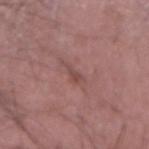follow-up: catalogued during a skin exam; not biopsied | illumination: white-light | body site: the left forearm | acquisition: total-body-photography crop, ~15 mm field of view | subject: male, about 50 years old | automated lesion analysis: an area of roughly 2.5 mm² and a shape eccentricity near 0.9; border irregularity of about 5 on a 0–10 scale, internal color variation of about 0 on a 0–10 scale, and a peripheral color-asymmetry measure near 0; an automated nevus-likeness rating near 0 out of 100 and a detector confidence of about 80 out of 100 that the crop contains a lesion | lesion diameter: about 3 mm.